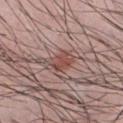| field | value |
|---|---|
| follow-up | total-body-photography surveillance lesion; no biopsy |
| lesion diameter | ~3.5 mm (longest diameter) |
| subject | male, about 30 years old |
| TBP lesion metrics | an average lesion color of about L≈48 a*≈22 b*≈24 (CIELAB), roughly 9 lightness units darker than nearby skin, and a lesion-to-skin contrast of about 7.5 (normalized; higher = more distinct); border irregularity of about 2.5 on a 0–10 scale, internal color variation of about 3 on a 0–10 scale, and peripheral color asymmetry of about 1; a nevus-likeness score of about 100/100 and a lesion-detection confidence of about 100/100 |
| body site | the chest |
| image source | total-body-photography crop, ~15 mm field of view |
| tile lighting | white-light |